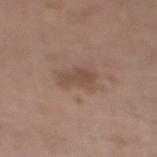biopsy_status: not biopsied; imaged during a skin examination
lesion_size:
  long_diameter_mm_approx: 4.0
image:
  source: total-body photography crop
  field_of_view_mm: 15
lighting: white-light
site: right lower leg
automated_metrics:
  area_mm2_approx: 5.5
  eccentricity: 0.9
  nevus_likeness_0_100: 0
patient:
  sex: male
  age_approx: 65